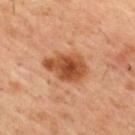Clinical impression: Part of a total-body skin-imaging series; this lesion was reviewed on a skin check and was not flagged for biopsy. Image and clinical context: On the upper back. A 15 mm close-up extracted from a 3D total-body photography capture. A male subject approximately 55 years of age.